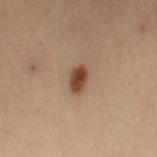Case summary:
- biopsy status: total-body-photography surveillance lesion; no biopsy
- lighting: cross-polarized
- location: the leg
- lesion diameter: about 3 mm
- patient: female, aged approximately 50
- acquisition: ~15 mm tile from a whole-body skin photo